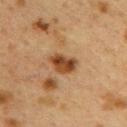Impression: Imaged during a routine full-body skin examination; the lesion was not biopsied and no histopathology is available. Context: Measured at roughly 3.5 mm in maximum diameter. The lesion is on the upper back. A roughly 15 mm field-of-view crop from a total-body skin photograph. Captured under cross-polarized illumination. A female patient aged 38–42. Automated image analysis of the tile measured a footprint of about 7 mm² and a shape eccentricity near 0.75. The analysis additionally found a mean CIELAB color near L≈37 a*≈19 b*≈31, about 12 CIELAB-L* units darker than the surrounding skin, and a lesion-to-skin contrast of about 10.5 (normalized; higher = more distinct). It also reported internal color variation of about 4.5 on a 0–10 scale and radial color variation of about 1.5.